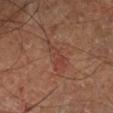Impression:
This lesion was catalogued during total-body skin photography and was not selected for biopsy.
Background:
The subject is a male approximately 65 years of age. Imaged with cross-polarized lighting. A close-up tile cropped from a whole-body skin photograph, about 15 mm across. Approximately 4.5 mm at its widest. From the leg.